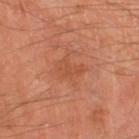This is a cross-polarized tile.
The lesion's longest dimension is about 3.5 mm.
A 15 mm close-up tile from a total-body photography series done for melanoma screening.
A male subject, approximately 65 years of age.
Located on the leg.